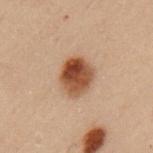Q: Was a biopsy performed?
A: imaged on a skin check; not biopsied
Q: Who is the patient?
A: male, about 55 years old
Q: How was the tile lit?
A: cross-polarized illumination
Q: Lesion location?
A: the left upper arm
Q: What kind of image is this?
A: 15 mm crop, total-body photography
Q: What is the lesion's diameter?
A: ≈4 mm